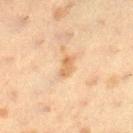Part of a total-body skin-imaging series; this lesion was reviewed on a skin check and was not flagged for biopsy.
The tile uses cross-polarized illumination.
The patient is a female aged 38–42.
Automated image analysis of the tile measured a footprint of about 3.5 mm² and an eccentricity of roughly 0.85.
The recorded lesion diameter is about 2.5 mm.
A roughly 15 mm field-of-view crop from a total-body skin photograph.
On the right lower leg.